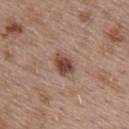The lesion was photographed on a routine skin check and not biopsied; there is no pathology result. The lesion's longest dimension is about 3 mm. The lesion is on the upper back. Automated image analysis of the tile measured a lesion area of about 5.5 mm², an outline eccentricity of about 0.65 (0 = round, 1 = elongated), and a symmetry-axis asymmetry near 0.25. The analysis additionally found a lesion color around L≈45 a*≈21 b*≈25 in CIELAB and a lesion-to-skin contrast of about 10 (normalized; higher = more distinct). It also reported border irregularity of about 2 on a 0–10 scale. A close-up tile cropped from a whole-body skin photograph, about 15 mm across. The patient is a female about 40 years old. This is a white-light tile.